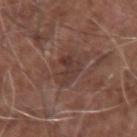Imaged during a routine full-body skin examination; the lesion was not biopsied and no histopathology is available. An algorithmic analysis of the crop reported a footprint of about 8 mm², an eccentricity of roughly 0.55, and two-axis asymmetry of about 0.35. It also reported an average lesion color of about L≈37 a*≈19 b*≈22 (CIELAB) and a normalized border contrast of about 6. The software also gave a border-irregularity index near 4/10, a within-lesion color-variation index near 3.5/10, and peripheral color asymmetry of about 1. It also reported a nevus-likeness score of about 0/100 and lesion-presence confidence of about 100/100. A 15 mm crop from a total-body photograph taken for skin-cancer surveillance. The lesion is located on the right forearm. The recorded lesion diameter is about 3.5 mm. Captured under white-light illumination. A male patient in their mid- to late 70s.